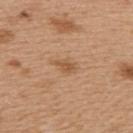biopsy status — catalogued during a skin exam; not biopsied | subject — female, aged 38–42 | lesion diameter — about 2.5 mm | image-analysis metrics — a footprint of about 3.5 mm², a shape eccentricity near 0.8, and a shape-asymmetry score of about 0.3 (0 = symmetric); an average lesion color of about L≈55 a*≈21 b*≈35 (CIELAB), roughly 8 lightness units darker than nearby skin, and a normalized lesion–skin contrast near 6; lesion-presence confidence of about 100/100 | site — the back | imaging modality — ~15 mm tile from a whole-body skin photo | tile lighting — white-light illumination.